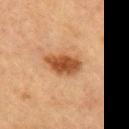Q: Was this lesion biopsied?
A: catalogued during a skin exam; not biopsied
Q: What lighting was used for the tile?
A: cross-polarized illumination
Q: Lesion size?
A: ≈4.5 mm
Q: How was this image acquired?
A: total-body-photography crop, ~15 mm field of view
Q: Patient demographics?
A: female, approximately 60 years of age
Q: Where on the body is the lesion?
A: the chest